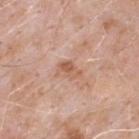No biopsy was performed on this lesion — it was imaged during a full skin examination and was not determined to be concerning. The recorded lesion diameter is about 3 mm. From the upper back. A lesion tile, about 15 mm wide, cut from a 3D total-body photograph. Captured under white-light illumination. A male subject approximately 50 years of age.